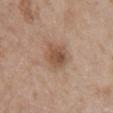Impression: Part of a total-body skin-imaging series; this lesion was reviewed on a skin check and was not flagged for biopsy. Image and clinical context: Cropped from a whole-body photographic skin survey; the tile spans about 15 mm. Captured under white-light illumination. Approximately 3.5 mm at its widest. The lesion is located on the chest. A female patient in their mid- to late 70s.